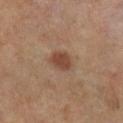Recorded during total-body skin imaging; not selected for excision or biopsy.
Cropped from a total-body skin-imaging series; the visible field is about 15 mm.
Longest diameter approximately 3 mm.
Automated image analysis of the tile measured an eccentricity of roughly 0.6 and a shape-asymmetry score of about 0.2 (0 = symmetric). It also reported a lesion color around L≈43 a*≈19 b*≈28 in CIELAB, about 10 CIELAB-L* units darker than the surrounding skin, and a lesion-to-skin contrast of about 8 (normalized; higher = more distinct). The software also gave a border-irregularity rating of about 1.5/10 and a within-lesion color-variation index near 1.5/10.
A female patient aged approximately 65.
Located on the left leg.
This is a cross-polarized tile.